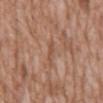follow-up — no biopsy performed (imaged during a skin exam)
acquisition — 15 mm crop, total-body photography
lighting — white-light
automated lesion analysis — an eccentricity of roughly 0.9; border irregularity of about 2 on a 0–10 scale and a color-variation rating of about 0/10
lesion diameter — ~2.5 mm (longest diameter)
anatomic site — the abdomen
subject — male, roughly 60 years of age